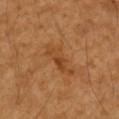Impression: This lesion was catalogued during total-body skin photography and was not selected for biopsy. Acquisition and patient details: The patient is a female aged 68 to 72. Automated image analysis of the tile measured an outline eccentricity of about 0.95 (0 = round, 1 = elongated) and a symmetry-axis asymmetry near 0.35. The software also gave about 7 CIELAB-L* units darker than the surrounding skin and a normalized lesion–skin contrast near 5.5. A lesion tile, about 15 mm wide, cut from a 3D total-body photograph. The lesion is on the arm.